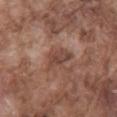No biopsy was performed on this lesion — it was imaged during a full skin examination and was not determined to be concerning. The lesion is located on the back. A male patient, approximately 75 years of age. The total-body-photography lesion software estimated a mean CIELAB color near L≈44 a*≈20 b*≈25 and a lesion–skin lightness drop of about 8. This image is a 15 mm lesion crop taken from a total-body photograph.